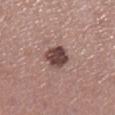Recorded during total-body skin imaging; not selected for excision or biopsy.
Imaged with white-light lighting.
Located on the left lower leg.
A lesion tile, about 15 mm wide, cut from a 3D total-body photograph.
A female patient roughly 60 years of age.
Measured at roughly 3.5 mm in maximum diameter.